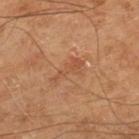  biopsy_status: not biopsied; imaged during a skin examination
  image:
    source: total-body photography crop
    field_of_view_mm: 15
  patient:
    sex: male
    age_approx: 65
  site: right lower leg